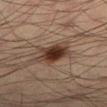workup — catalogued during a skin exam; not biopsied | image-analysis metrics — a border-irregularity index near 3.5/10, a color-variation rating of about 5.5/10, and a peripheral color-asymmetry measure near 1.5; an automated nevus-likeness rating near 100 out of 100 and a lesion-detection confidence of about 100/100 | image — 15 mm crop, total-body photography | lighting — cross-polarized | patient — male, in their mid-30s | diameter — ~4.5 mm (longest diameter) | body site — the left lower leg.